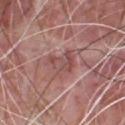About 3.5 mm across. A 15 mm close-up tile from a total-body photography series done for melanoma screening. The tile uses white-light illumination. The patient is a male about 65 years old. The lesion is on the chest.